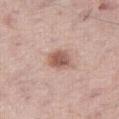{"biopsy_status": "not biopsied; imaged during a skin examination", "site": "right thigh", "image": {"source": "total-body photography crop", "field_of_view_mm": 15}, "patient": {"sex": "male", "age_approx": 75}, "lighting": "white-light", "automated_metrics": {"area_mm2_approx": 6.0, "eccentricity": 0.6}}